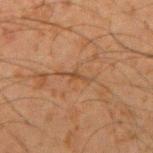The lesion was photographed on a routine skin check and not biopsied; there is no pathology result. A close-up tile cropped from a whole-body skin photograph, about 15 mm across. A male subject in their mid- to late 30s. Imaged with cross-polarized lighting. Longest diameter approximately 3 mm. An algorithmic analysis of the crop reported a shape eccentricity near 0.95. The software also gave a lesion color around L≈36 a*≈16 b*≈28 in CIELAB, a lesion–skin lightness drop of about 6, and a normalized lesion–skin contrast near 5.5. And it measured a border-irregularity index near 6/10 and a color-variation rating of about 0/10. The software also gave lesion-presence confidence of about 50/100. On the right upper arm.